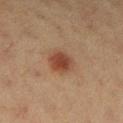notes — catalogued during a skin exam; not biopsied | automated metrics — an eccentricity of roughly 0.35 and a shape-asymmetry score of about 0.2 (0 = symmetric); border irregularity of about 1.5 on a 0–10 scale, a within-lesion color-variation index near 3/10, and peripheral color asymmetry of about 1; a nevus-likeness score of about 100/100 and a detector confidence of about 100 out of 100 that the crop contains a lesion | subject — female, roughly 50 years of age | lesion size — about 3 mm | location — the right lower leg | image — total-body-photography crop, ~15 mm field of view | tile lighting — cross-polarized.